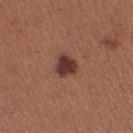Q: Was this lesion biopsied?
A: imaged on a skin check; not biopsied
Q: Who is the patient?
A: female, about 30 years old
Q: Lesion location?
A: the right thigh
Q: How was this image acquired?
A: ~15 mm crop, total-body skin-cancer survey
Q: Automated lesion metrics?
A: a footprint of about 5.5 mm², an outline eccentricity of about 0.55 (0 = round, 1 = elongated), and two-axis asymmetry of about 0.3; a border-irregularity rating of about 2.5/10 and radial color variation of about 1